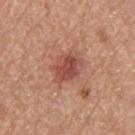Impression: Part of a total-body skin-imaging series; this lesion was reviewed on a skin check and was not flagged for biopsy. Image and clinical context: A 15 mm close-up tile from a total-body photography series done for melanoma screening. A male subject aged 53 to 57. The tile uses white-light illumination. The lesion is on the upper back. About 3.5 mm across.